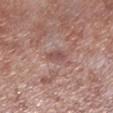biopsy status: total-body-photography surveillance lesion; no biopsy
lighting: white-light
acquisition: ~15 mm crop, total-body skin-cancer survey
subject: male, approximately 55 years of age
location: the left lower leg